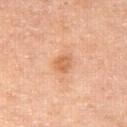No biopsy was performed on this lesion — it was imaged during a full skin examination and was not determined to be concerning.
A female subject about 55 years old.
Located on the right thigh.
A lesion tile, about 15 mm wide, cut from a 3D total-body photograph.